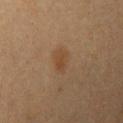follow-up=imaged on a skin check; not biopsied
image-analysis metrics=a border-irregularity index near 2/10, a within-lesion color-variation index near 1/10, and radial color variation of about 0.5
lighting=cross-polarized
subject=female, aged approximately 40
acquisition=total-body-photography crop, ~15 mm field of view
anatomic site=the left upper arm
lesion size=≈3 mm